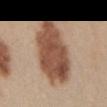Clinical impression: Captured during whole-body skin photography for melanoma surveillance; the lesion was not biopsied. Context: Approximately 9.5 mm at its widest. The subject is a male aged 28 to 32. Captured under white-light illumination. The lesion is located on the abdomen. Cropped from a whole-body photographic skin survey; the tile spans about 15 mm.